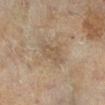Impression: The lesion was photographed on a routine skin check and not biopsied; there is no pathology result. Context: A 15 mm close-up extracted from a 3D total-body photography capture. Automated tile analysis of the lesion measured border irregularity of about 4 on a 0–10 scale and peripheral color asymmetry of about 0.5. A female subject, in their 60s. This is a cross-polarized tile. On the right lower leg.